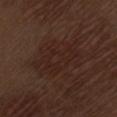Notes:
– workup · imaged on a skin check; not biopsied
– TBP lesion metrics · a lesion color around L≈23 a*≈17 b*≈20 in CIELAB, roughly 4 lightness units darker than nearby skin, and a normalized lesion–skin contrast near 4.5; internal color variation of about 2.5 on a 0–10 scale and radial color variation of about 1
– subject · male, aged approximately 70
– body site · the left thigh
– illumination · white-light illumination
– imaging modality · 15 mm crop, total-body photography
– size · ≈7 mm